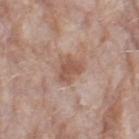Impression: No biopsy was performed on this lesion — it was imaged during a full skin examination and was not determined to be concerning. Context: The tile uses white-light illumination. The lesion-visualizer software estimated about 10 CIELAB-L* units darker than the surrounding skin. It also reported a border-irregularity rating of about 3.5/10, a within-lesion color-variation index near 2/10, and a peripheral color-asymmetry measure near 0.5. It also reported a lesion-detection confidence of about 100/100. About 3 mm across. A female subject, aged 68–72. Cropped from a whole-body photographic skin survey; the tile spans about 15 mm. The lesion is located on the left thigh.